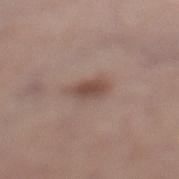Recorded during total-body skin imaging; not selected for excision or biopsy. A 15 mm crop from a total-body photograph taken for skin-cancer surveillance. On the left lower leg. Automated image analysis of the tile measured an area of roughly 5 mm², an eccentricity of roughly 0.85, and two-axis asymmetry of about 0.2. It also reported a classifier nevus-likeness of about 90/100 and a lesion-detection confidence of about 100/100. A female subject aged 63–67. The lesion's longest dimension is about 3.5 mm.